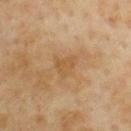The lesion was tiled from a total-body skin photograph and was not biopsied.